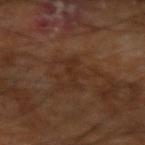workup = imaged on a skin check; not biopsied
patient = male, about 60 years old
imaging modality = total-body-photography crop, ~15 mm field of view
site = the left arm